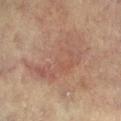Captured during whole-body skin photography for melanoma surveillance; the lesion was not biopsied.
The lesion is located on the left lower leg.
A female patient roughly 80 years of age.
A 15 mm close-up extracted from a 3D total-body photography capture.